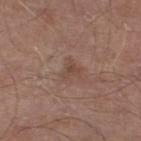No biopsy was performed on this lesion — it was imaged during a full skin examination and was not determined to be concerning.
A male patient, in their 70s.
Cropped from a total-body skin-imaging series; the visible field is about 15 mm.
The lesion is on the left lower leg.